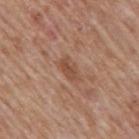workup: no biopsy performed (imaged during a skin exam); image source: ~15 mm tile from a whole-body skin photo; patient: male, aged 58–62; site: the mid back; lesion size: ≈3 mm; tile lighting: white-light.